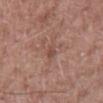follow-up: imaged on a skin check; not biopsied
lesion diameter: ≈2.5 mm
image source: ~15 mm tile from a whole-body skin photo
illumination: white-light illumination
anatomic site: the mid back
patient: male, roughly 55 years of age
automated lesion analysis: an area of roughly 3 mm², an outline eccentricity of about 0.85 (0 = round, 1 = elongated), and a shape-asymmetry score of about 0.3 (0 = symmetric); a classifier nevus-likeness of about 0/100 and a lesion-detection confidence of about 95/100